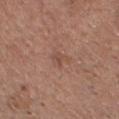<case>
<biopsy_status>not biopsied; imaged during a skin examination</biopsy_status>
<automated_metrics>
  <cielab_L>49</cielab_L>
  <cielab_a>20</cielab_a>
  <cielab_b>27</cielab_b>
  <vs_skin_darker_L>6.0</vs_skin_darker_L>
  <vs_skin_contrast_norm>4.5</vs_skin_contrast_norm>
  <border_irregularity_0_10>5.0</border_irregularity_0_10>
  <color_variation_0_10>1.5</color_variation_0_10>
  <peripheral_color_asymmetry>0.5</peripheral_color_asymmetry>
  <nevus_likeness_0_100>0</nevus_likeness_0_100>
  <lesion_detection_confidence_0_100>100</lesion_detection_confidence_0_100>
</automated_metrics>
<site>head or neck</site>
<lighting>white-light</lighting>
<patient>
  <sex>male</sex>
  <age_approx>65</age_approx>
</patient>
<image>
  <source>total-body photography crop</source>
  <field_of_view_mm>15</field_of_view_mm>
</image>
</case>